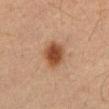Case summary:
* biopsy status · imaged on a skin check; not biopsied
* lesion size · about 4 mm
* site · the mid back
* imaging modality · ~15 mm tile from a whole-body skin photo
* image-analysis metrics · an area of roughly 8.5 mm², an eccentricity of roughly 0.7, and a symmetry-axis asymmetry near 0.2; a detector confidence of about 100 out of 100 that the crop contains a lesion
* patient · male, aged 63–67
* tile lighting · cross-polarized